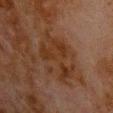Assessment: Part of a total-body skin-imaging series; this lesion was reviewed on a skin check and was not flagged for biopsy. Clinical summary: The lesion is located on the chest. Cropped from a total-body skin-imaging series; the visible field is about 15 mm. Longest diameter approximately 6 mm. Captured under cross-polarized illumination. The patient is a male aged 78 to 82.